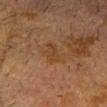A male subject roughly 75 years of age. An algorithmic analysis of the crop reported a within-lesion color-variation index near 1/10 and a peripheral color-asymmetry measure near 0. The analysis additionally found a lesion-detection confidence of about 100/100. On the head or neck. Captured under cross-polarized illumination. Approximately 3 mm at its widest. A roughly 15 mm field-of-view crop from a total-body skin photograph.